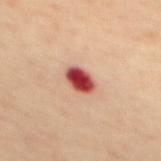Q: Was this lesion biopsied?
A: total-body-photography surveillance lesion; no biopsy
Q: Illumination type?
A: cross-polarized
Q: Automated lesion metrics?
A: an area of roughly 6 mm², an eccentricity of roughly 0.75, and a shape-asymmetry score of about 0.15 (0 = symmetric); a border-irregularity index near 1.5/10 and radial color variation of about 1.5; a classifier nevus-likeness of about 0/100 and a detector confidence of about 100 out of 100 that the crop contains a lesion
Q: What is the imaging modality?
A: ~15 mm crop, total-body skin-cancer survey
Q: What is the lesion's diameter?
A: ~3 mm (longest diameter)
Q: What is the anatomic site?
A: the mid back
Q: What are the patient's age and sex?
A: female, aged around 70